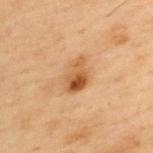A male subject aged 53 to 57.
Longest diameter approximately 3.5 mm.
Cropped from a total-body skin-imaging series; the visible field is about 15 mm.
From the upper back.
Automated tile analysis of the lesion measured a footprint of about 7 mm², an outline eccentricity of about 0.7 (0 = round, 1 = elongated), and a symmetry-axis asymmetry near 0.3. The software also gave a lesion–skin lightness drop of about 10 and a lesion-to-skin contrast of about 8.5 (normalized; higher = more distinct). It also reported a classifier nevus-likeness of about 90/100.
Imaged with cross-polarized lighting.